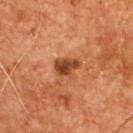– follow-up · imaged on a skin check; not biopsied
– imaging modality · total-body-photography crop, ~15 mm field of view
– size · ~3 mm (longest diameter)
– subject · male, roughly 55 years of age
– automated lesion analysis · a lesion area of about 5 mm², an outline eccentricity of about 0.7 (0 = round, 1 = elongated), and a shape-asymmetry score of about 0.25 (0 = symmetric); an average lesion color of about L≈41 a*≈25 b*≈35 (CIELAB), a lesion–skin lightness drop of about 13, and a lesion-to-skin contrast of about 10 (normalized; higher = more distinct); a classifier nevus-likeness of about 65/100 and a lesion-detection confidence of about 100/100
– lighting · cross-polarized
– site · the chest